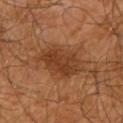Imaged during a routine full-body skin examination; the lesion was not biopsied and no histopathology is available. A 15 mm close-up extracted from a 3D total-body photography capture. Imaged with cross-polarized lighting. A male patient in their mid-60s. Approximately 5.5 mm at its widest. The total-body-photography lesion software estimated border irregularity of about 3 on a 0–10 scale, a within-lesion color-variation index near 3.5/10, and radial color variation of about 1. The lesion is located on the right upper arm.